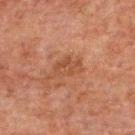{
  "biopsy_status": "not biopsied; imaged during a skin examination",
  "automated_metrics": {
    "area_mm2_approx": 6.0,
    "eccentricity": 0.8,
    "shape_asymmetry": 0.25
  },
  "lesion_size": {
    "long_diameter_mm_approx": 3.5
  },
  "patient": {
    "sex": "male",
    "age_approx": 60
  },
  "lighting": "cross-polarized",
  "site": "back",
  "image": {
    "source": "total-body photography crop",
    "field_of_view_mm": 15
  }
}